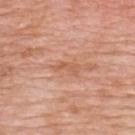Assessment:
This lesion was catalogued during total-body skin photography and was not selected for biopsy.
Clinical summary:
Automated image analysis of the tile measured a lesion color around L≈59 a*≈24 b*≈34 in CIELAB, a lesion–skin lightness drop of about 7, and a normalized lesion–skin contrast near 5.5. And it measured a nevus-likeness score of about 0/100 and a lesion-detection confidence of about 80/100. Longest diameter approximately 3 mm. A lesion tile, about 15 mm wide, cut from a 3D total-body photograph. The lesion is on the upper back. The subject is a female aged 68 to 72. Captured under white-light illumination.